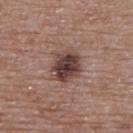Clinical impression:
Recorded during total-body skin imaging; not selected for excision or biopsy.
Clinical summary:
The lesion-visualizer software estimated an area of roughly 10 mm², a shape eccentricity near 0.45, and a symmetry-axis asymmetry near 0.15. The software also gave an average lesion color of about L≈40 a*≈18 b*≈21 (CIELAB). The tile uses white-light illumination. The patient is a female in their mid- to late 60s. A region of skin cropped from a whole-body photographic capture, roughly 15 mm wide. The lesion is located on the back.